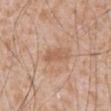biopsy_status: not biopsied; imaged during a skin examination